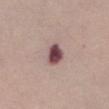Impression: Recorded during total-body skin imaging; not selected for excision or biopsy. Clinical summary: About 3 mm across. Imaged with white-light lighting. The lesion is on the chest. The patient is a female aged 63–67. The total-body-photography lesion software estimated a lesion area of about 5.5 mm², a shape eccentricity near 0.65, and a symmetry-axis asymmetry near 0.2. And it measured a border-irregularity rating of about 1.5/10 and internal color variation of about 4 on a 0–10 scale. The analysis additionally found a detector confidence of about 100 out of 100 that the crop contains a lesion. A 15 mm close-up tile from a total-body photography series done for melanoma screening.